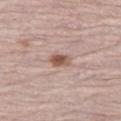<tbp_lesion>
<biopsy_status>not biopsied; imaged during a skin examination</biopsy_status>
<image>
  <source>total-body photography crop</source>
  <field_of_view_mm>15</field_of_view_mm>
</image>
<lesion_size>
  <long_diameter_mm_approx>2.5</long_diameter_mm_approx>
</lesion_size>
<site>left thigh</site>
<lighting>white-light</lighting>
<patient>
  <sex>female</sex>
  <age_approx>65</age_approx>
</patient>
<automated_metrics>
  <border_irregularity_0_10>2.0</border_irregularity_0_10>
  <nevus_likeness_0_100>95</nevus_likeness_0_100>
  <lesion_detection_confidence_0_100>100</lesion_detection_confidence_0_100>
</automated_metrics>
</tbp_lesion>